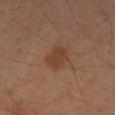Recorded during total-body skin imaging; not selected for excision or biopsy. A 15 mm close-up extracted from a 3D total-body photography capture. Longest diameter approximately 3 mm. The lesion is located on the arm. Automated image analysis of the tile measured an average lesion color of about L≈40 a*≈21 b*≈30 (CIELAB), roughly 7 lightness units darker than nearby skin, and a normalized lesion–skin contrast near 7. It also reported a border-irregularity rating of about 2/10 and internal color variation of about 2 on a 0–10 scale. The patient is a female in their 40s.